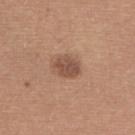Part of a total-body skin-imaging series; this lesion was reviewed on a skin check and was not flagged for biopsy. Automated tile analysis of the lesion measured a shape eccentricity near 0.7 and a symmetry-axis asymmetry near 0.2. The software also gave roughly 11 lightness units darker than nearby skin and a lesion-to-skin contrast of about 8 (normalized; higher = more distinct). And it measured a nevus-likeness score of about 50/100 and a lesion-detection confidence of about 100/100. A female subject, aged approximately 55. Captured under white-light illumination. On the upper back. A region of skin cropped from a whole-body photographic capture, roughly 15 mm wide. Measured at roughly 3.5 mm in maximum diameter.